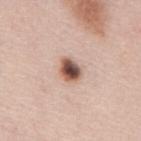Captured during whole-body skin photography for melanoma surveillance; the lesion was not biopsied.
About 3 mm across.
The patient is a male roughly 45 years of age.
A 15 mm close-up tile from a total-body photography series done for melanoma screening.
The tile uses white-light illumination.
Located on the mid back.